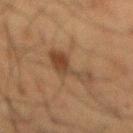Impression: This lesion was catalogued during total-body skin photography and was not selected for biopsy. Context: A 15 mm close-up extracted from a 3D total-body photography capture. This is a cross-polarized tile. On the back. Automated image analysis of the tile measured an area of roughly 8.5 mm², an outline eccentricity of about 0.85 (0 = round, 1 = elongated), and a shape-asymmetry score of about 0.65 (0 = symmetric). The analysis additionally found a within-lesion color-variation index near 3.5/10 and radial color variation of about 1. And it measured a classifier nevus-likeness of about 50/100 and a detector confidence of about 100 out of 100 that the crop contains a lesion. Longest diameter approximately 5 mm. A male patient approximately 60 years of age.